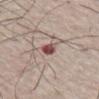Captured during whole-body skin photography for melanoma surveillance; the lesion was not biopsied. A lesion tile, about 15 mm wide, cut from a 3D total-body photograph. On the chest. The total-body-photography lesion software estimated a lesion color around L≈49 a*≈23 b*≈20 in CIELAB and a normalized lesion–skin contrast near 11. The analysis additionally found border irregularity of about 3 on a 0–10 scale, a within-lesion color-variation index near 4/10, and radial color variation of about 1.5. The tile uses white-light illumination. The subject is a male aged approximately 65.